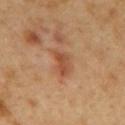Acquisition and patient details:
A male patient approximately 50 years of age. Longest diameter approximately 3.5 mm. This is a cross-polarized tile. Automated tile analysis of the lesion measured a lesion color around L≈49 a*≈25 b*≈34 in CIELAB and about 10 CIELAB-L* units darker than the surrounding skin. And it measured a border-irregularity rating of about 3.5/10, a within-lesion color-variation index near 2/10, and peripheral color asymmetry of about 0.5. It also reported an automated nevus-likeness rating near 0 out of 100. Cropped from a total-body skin-imaging series; the visible field is about 15 mm. From the mid back.